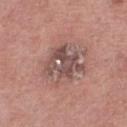Q: Is there a histopathology result?
A: no biopsy performed (imaged during a skin exam)
Q: How was this image acquired?
A: ~15 mm crop, total-body skin-cancer survey
Q: Patient demographics?
A: female, roughly 70 years of age
Q: Where on the body is the lesion?
A: the leg